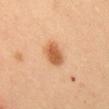The subject is a female aged 38–42. The lesion is located on the chest. Captured under cross-polarized illumination. Cropped from a total-body skin-imaging series; the visible field is about 15 mm.